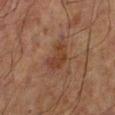Captured during whole-body skin photography for melanoma surveillance; the lesion was not biopsied. A male subject aged approximately 70. On the left lower leg. Cropped from a whole-body photographic skin survey; the tile spans about 15 mm.